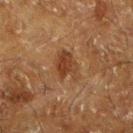Impression: Recorded during total-body skin imaging; not selected for excision or biopsy. Acquisition and patient details: The lesion-visualizer software estimated an area of roughly 7.5 mm², a shape eccentricity near 0.75, and a shape-asymmetry score of about 0.35 (0 = symmetric). And it measured a border-irregularity index near 4/10 and a color-variation rating of about 2.5/10. The analysis additionally found a classifier nevus-likeness of about 20/100 and a lesion-detection confidence of about 100/100. A male subject in their 60s. The lesion is located on the left lower leg. A 15 mm close-up tile from a total-body photography series done for melanoma screening. Captured under cross-polarized illumination.